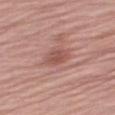notes: imaged on a skin check; not biopsied
subject: female, approximately 70 years of age
tile lighting: white-light
anatomic site: the right thigh
image source: total-body-photography crop, ~15 mm field of view
TBP lesion metrics: a lesion area of about 4.5 mm², a shape eccentricity near 0.8, and a shape-asymmetry score of about 0.25 (0 = symmetric); a lesion color around L≈52 a*≈24 b*≈25 in CIELAB, about 9 CIELAB-L* units darker than the surrounding skin, and a lesion-to-skin contrast of about 6.5 (normalized; higher = more distinct); a lesion-detection confidence of about 100/100